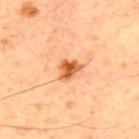Notes:
- follow-up · catalogued during a skin exam; not biopsied
- site · the back
- imaging modality · total-body-photography crop, ~15 mm field of view
- subject · male, aged 58–62
- lighting · cross-polarized
- TBP lesion metrics · a footprint of about 4.5 mm², a shape eccentricity near 0.6, and a shape-asymmetry score of about 0.3 (0 = symmetric); roughly 16 lightness units darker than nearby skin and a lesion-to-skin contrast of about 10 (normalized; higher = more distinct); a nevus-likeness score of about 90/100